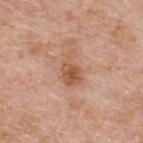Notes:
- patient · male, in their mid-70s
- imaging modality · total-body-photography crop, ~15 mm field of view
- site · the upper back